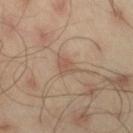biopsy status: imaged on a skin check; not biopsied
imaging modality: 15 mm crop, total-body photography
lesion size: ~3 mm (longest diameter)
automated lesion analysis: an automated nevus-likeness rating near 0 out of 100 and a lesion-detection confidence of about 100/100
body site: the right thigh
illumination: cross-polarized illumination
patient: male, aged approximately 45